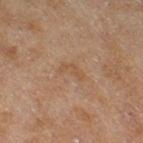Clinical impression:
Imaged during a routine full-body skin examination; the lesion was not biopsied and no histopathology is available.
Context:
Located on the right thigh. Imaged with cross-polarized lighting. A lesion tile, about 15 mm wide, cut from a 3D total-body photograph. The subject is a female about 60 years old. About 3 mm across.